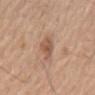Impression:
This lesion was catalogued during total-body skin photography and was not selected for biopsy.
Context:
The lesion is located on the mid back. A 15 mm crop from a total-body photograph taken for skin-cancer surveillance. About 3 mm across. The total-body-photography lesion software estimated an area of roughly 5 mm², an outline eccentricity of about 0.65 (0 = round, 1 = elongated), and two-axis asymmetry of about 0.35. And it measured a mean CIELAB color near L≈55 a*≈20 b*≈30, about 10 CIELAB-L* units darker than the surrounding skin, and a normalized lesion–skin contrast near 6.5. The patient is a male roughly 65 years of age. The tile uses white-light illumination.